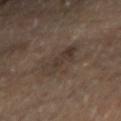<tbp_lesion>
  <biopsy_status>not biopsied; imaged during a skin examination</biopsy_status>
  <site>right forearm</site>
  <image>
    <source>total-body photography crop</source>
    <field_of_view_mm>15</field_of_view_mm>
  </image>
  <lighting>cross-polarized</lighting>
  <lesion_size>
    <long_diameter_mm_approx>4.0</long_diameter_mm_approx>
  </lesion_size>
  <automated_metrics>
    <area_mm2_approx>7.5</area_mm2_approx>
    <eccentricity>0.85</eccentricity>
    <shape_asymmetry>0.3</shape_asymmetry>
    <cielab_L>33</cielab_L>
    <cielab_a>11</cielab_a>
    <cielab_b>20</cielab_b>
    <vs_skin_darker_L>6.0</vs_skin_darker_L>
    <vs_skin_contrast_norm>6.5</vs_skin_contrast_norm>
    <border_irregularity_0_10>4.0</border_irregularity_0_10>
    <nevus_likeness_0_100>5</nevus_likeness_0_100>
    <lesion_detection_confidence_0_100>100</lesion_detection_confidence_0_100>
  </automated_metrics>
  <patient>
    <sex>male</sex>
    <age_approx>65</age_approx>
  </patient>
</tbp_lesion>